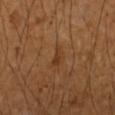Findings:
– follow-up: catalogued during a skin exam; not biopsied
– lesion diameter: about 3 mm
– patient: male, roughly 55 years of age
– image: 15 mm crop, total-body photography
– site: the right forearm
– tile lighting: cross-polarized illumination